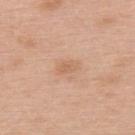The lesion was photographed on a routine skin check and not biopsied; there is no pathology result. Approximately 2.5 mm at its widest. On the upper back. The subject is a male aged 58 to 62. This is a white-light tile. The total-body-photography lesion software estimated a footprint of about 3.5 mm² and an outline eccentricity of about 0.85 (0 = round, 1 = elongated). The software also gave a border-irregularity rating of about 2.5/10 and a color-variation rating of about 1.5/10. Cropped from a total-body skin-imaging series; the visible field is about 15 mm.